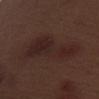notes: catalogued during a skin exam; not biopsied | TBP lesion metrics: two-axis asymmetry of about 0.45; a lesion color around L≈23 a*≈17 b*≈18 in CIELAB, about 6 CIELAB-L* units darker than the surrounding skin, and a normalized lesion–skin contrast near 7 | illumination: white-light | acquisition: 15 mm crop, total-body photography | anatomic site: the left upper arm | diameter: about 9 mm | patient: male, aged around 70.